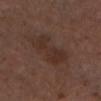| feature | finding |
|---|---|
| notes | imaged on a skin check; not biopsied |
| patient | male, approximately 75 years of age |
| imaging modality | ~15 mm crop, total-body skin-cancer survey |
| diameter | ~5 mm (longest diameter) |
| body site | the head or neck |